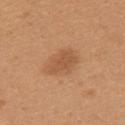Clinical impression:
No biopsy was performed on this lesion — it was imaged during a full skin examination and was not determined to be concerning.
Clinical summary:
From the back. Measured at roughly 4 mm in maximum diameter. This is a white-light tile. The patient is a female about 25 years old. A lesion tile, about 15 mm wide, cut from a 3D total-body photograph.